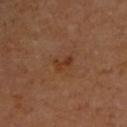follow-up = catalogued during a skin exam; not biopsied
tile lighting = cross-polarized illumination
patient = male, aged 63 to 67
location = the right upper arm
image source = ~15 mm crop, total-body skin-cancer survey